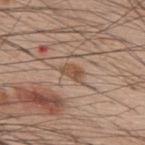Q: Was this lesion biopsied?
A: no biopsy performed (imaged during a skin exam)
Q: What did automated image analysis measure?
A: a lesion area of about 3.5 mm², a shape eccentricity near 0.8, and a shape-asymmetry score of about 0.25 (0 = symmetric); a lesion color around L≈51 a*≈19 b*≈30 in CIELAB, a lesion–skin lightness drop of about 9, and a lesion-to-skin contrast of about 7.5 (normalized; higher = more distinct); a classifier nevus-likeness of about 0/100 and a detector confidence of about 100 out of 100 that the crop contains a lesion
Q: What is the imaging modality?
A: ~15 mm tile from a whole-body skin photo
Q: How was the tile lit?
A: white-light
Q: Who is the patient?
A: male, approximately 60 years of age
Q: Lesion location?
A: the back
Q: How large is the lesion?
A: ~2.5 mm (longest diameter)